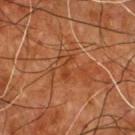Notes:
– follow-up · imaged on a skin check; not biopsied
– subject · male, aged 53 to 57
– automated metrics · border irregularity of about 6 on a 0–10 scale, a within-lesion color-variation index near 0/10, and a peripheral color-asymmetry measure near 0
– anatomic site · the chest
– lesion diameter · ≈3 mm
– tile lighting · cross-polarized illumination
– image source · 15 mm crop, total-body photography